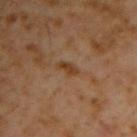biopsy status: no biopsy performed (imaged during a skin exam) | body site: the right upper arm | subject: male, aged 58 to 62 | automated lesion analysis: a lesion area of about 2.5 mm², an outline eccentricity of about 0.9 (0 = round, 1 = elongated), and a shape-asymmetry score of about 0.3 (0 = symmetric); a lesion color around L≈36 a*≈19 b*≈31 in CIELAB; a nevus-likeness score of about 5/100 and lesion-presence confidence of about 100/100 | lighting: cross-polarized | lesion size: ≈2.5 mm | imaging modality: total-body-photography crop, ~15 mm field of view.